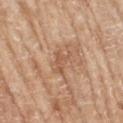Case summary:
* tile lighting: white-light illumination
* automated metrics: a footprint of about 4 mm² and a shape eccentricity near 0.8; a border-irregularity rating of about 6/10, internal color variation of about 1 on a 0–10 scale, and a peripheral color-asymmetry measure near 0.5; an automated nevus-likeness rating near 0 out of 100 and a lesion-detection confidence of about 95/100
* lesion size: ~3 mm (longest diameter)
* image: ~15 mm crop, total-body skin-cancer survey
* patient: female, roughly 75 years of age
* location: the right upper arm